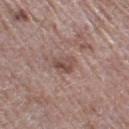No biopsy was performed on this lesion — it was imaged during a full skin examination and was not determined to be concerning.
Approximately 2.5 mm at its widest.
A male subject aged 68 to 72.
The lesion is on the left thigh.
A 15 mm close-up extracted from a 3D total-body photography capture.
Automated tile analysis of the lesion measured a footprint of about 4.5 mm², a shape eccentricity near 0.65, and a symmetry-axis asymmetry near 0.3. The analysis additionally found an average lesion color of about L≈48 a*≈19 b*≈22 (CIELAB), about 9 CIELAB-L* units darker than the surrounding skin, and a normalized border contrast of about 7. And it measured a nevus-likeness score of about 5/100.